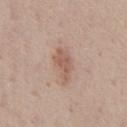Notes:
• notes — imaged on a skin check; not biopsied
• anatomic site — the chest
• lesion diameter — ~4 mm (longest diameter)
• imaging modality — ~15 mm tile from a whole-body skin photo
• tile lighting — white-light illumination
• automated metrics — a shape eccentricity near 0.85 and two-axis asymmetry of about 0.25; a border-irregularity rating of about 3.5/10, a within-lesion color-variation index near 2/10, and radial color variation of about 0.5; a nevus-likeness score of about 35/100
• patient — male, aged 38–42